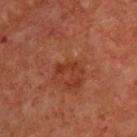The lesion was photographed on a routine skin check and not biopsied; there is no pathology result. This is a cross-polarized tile. A male subject, about 65 years old. About 3 mm across. A close-up tile cropped from a whole-body skin photograph, about 15 mm across. On the front of the torso.